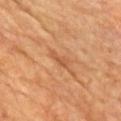Q: Was this lesion biopsied?
A: imaged on a skin check; not biopsied
Q: Where on the body is the lesion?
A: the chest
Q: How was this image acquired?
A: total-body-photography crop, ~15 mm field of view
Q: What is the lesion's diameter?
A: ≈3 mm
Q: Who is the patient?
A: male, in their mid-80s or older
Q: What lighting was used for the tile?
A: cross-polarized
Q: Automated lesion metrics?
A: an outline eccentricity of about 0.9 (0 = round, 1 = elongated) and a symmetry-axis asymmetry near 0.3; a mean CIELAB color near L≈54 a*≈25 b*≈39, roughly 8 lightness units darker than nearby skin, and a normalized border contrast of about 5.5; an automated nevus-likeness rating near 0 out of 100